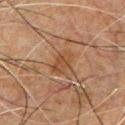<case>
<biopsy_status>not biopsied; imaged during a skin examination</biopsy_status>
<site>front of the torso</site>
<patient>
  <sex>male</sex>
  <age_approx>50</age_approx>
</patient>
<automated_metrics>
  <area_mm2_approx>6.0</area_mm2_approx>
  <eccentricity>0.75</eccentricity>
  <shape_asymmetry>0.25</shape_asymmetry>
  <nevus_likeness_0_100>0</nevus_likeness_0_100>
</automated_metrics>
<lesion_size>
  <long_diameter_mm_approx>3.0</long_diameter_mm_approx>
</lesion_size>
<lighting>cross-polarized</lighting>
<image>
  <source>total-body photography crop</source>
  <field_of_view_mm>15</field_of_view_mm>
</image>
</case>